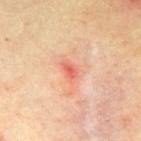biopsy status=catalogued during a skin exam; not biopsied | acquisition=15 mm crop, total-body photography | location=the abdomen | illumination=cross-polarized | patient=male, in their mid- to late 60s.